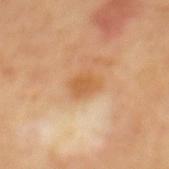follow-up = catalogued during a skin exam; not biopsied | TBP lesion metrics = roughly 9 lightness units darker than nearby skin; a within-lesion color-variation index near 2/10 and peripheral color asymmetry of about 1 | lesion diameter = ~3 mm (longest diameter) | patient = male, aged around 65 | location = the mid back | tile lighting = cross-polarized | acquisition = ~15 mm tile from a whole-body skin photo.